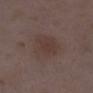Assessment:
Imaged during a routine full-body skin examination; the lesion was not biopsied and no histopathology is available.
Clinical summary:
The tile uses white-light illumination. A roughly 15 mm field-of-view crop from a total-body skin photograph. A female patient, aged around 30. From the right lower leg.